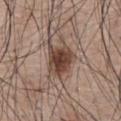Captured during whole-body skin photography for melanoma surveillance; the lesion was not biopsied. Approximately 4 mm at its widest. The tile uses white-light illumination. The lesion-visualizer software estimated a lesion area of about 11 mm², an eccentricity of roughly 0.45, and two-axis asymmetry of about 0.25. And it measured a mean CIELAB color near L≈43 a*≈17 b*≈24, a lesion–skin lightness drop of about 14, and a lesion-to-skin contrast of about 11 (normalized; higher = more distinct). And it measured a color-variation rating of about 5/10. Located on the abdomen. A region of skin cropped from a whole-body photographic capture, roughly 15 mm wide. The subject is a male in their mid- to late 70s.